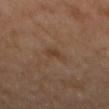Case summary:
* site: the mid back
* TBP lesion metrics: a lesion area of about 3.5 mm² and a symmetry-axis asymmetry near 0.4
* subject: male, aged around 65
* tile lighting: cross-polarized illumination
* image: ~15 mm tile from a whole-body skin photo